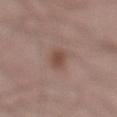The lesion was photographed on a routine skin check and not biopsied; there is no pathology result. A male patient, aged approximately 25. Longest diameter approximately 3.5 mm. This is a white-light tile. A 15 mm close-up extracted from a 3D total-body photography capture. On the left lower leg. The lesion-visualizer software estimated roughly 9 lightness units darker than nearby skin and a normalized border contrast of about 7.